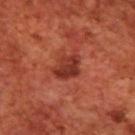biopsy_status: not biopsied; imaged during a skin examination
image:
  source: total-body photography crop
  field_of_view_mm: 15
lighting: cross-polarized
patient:
  sex: male
  age_approx: 70
lesion_size:
  long_diameter_mm_approx: 3.5
site: upper back